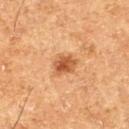workup = no biopsy performed (imaged during a skin exam)
lesion diameter = ≈3 mm
patient = male, aged 73 to 77
image = 15 mm crop, total-body photography
anatomic site = the left thigh
tile lighting = cross-polarized illumination